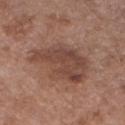No biopsy was performed on this lesion — it was imaged during a full skin examination and was not determined to be concerning.
From the chest.
The lesion's longest dimension is about 7 mm.
A female subject, roughly 80 years of age.
An algorithmic analysis of the crop reported border irregularity of about 4 on a 0–10 scale, a within-lesion color-variation index near 4.5/10, and radial color variation of about 1.5.
A close-up tile cropped from a whole-body skin photograph, about 15 mm across.
The tile uses white-light illumination.